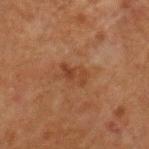Recorded during total-body skin imaging; not selected for excision or biopsy. A male subject about 75 years old. From the left forearm. The lesion's longest dimension is about 3 mm. Cropped from a whole-body photographic skin survey; the tile spans about 15 mm. Automated image analysis of the tile measured a lesion–skin lightness drop of about 6 and a lesion-to-skin contrast of about 6 (normalized; higher = more distinct). And it measured a border-irregularity index near 5/10, internal color variation of about 1 on a 0–10 scale, and radial color variation of about 0. It also reported an automated nevus-likeness rating near 0 out of 100 and a detector confidence of about 100 out of 100 that the crop contains a lesion.